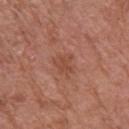<lesion>
<biopsy_status>not biopsied; imaged during a skin examination</biopsy_status>
<image>
  <source>total-body photography crop</source>
  <field_of_view_mm>15</field_of_view_mm>
</image>
<patient>
  <sex>female</sex>
  <age_approx>80</age_approx>
</patient>
<lighting>white-light</lighting>
<site>arm</site>
<lesion_size>
  <long_diameter_mm_approx>2.5</long_diameter_mm_approx>
</lesion_size>
</lesion>